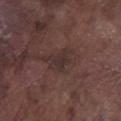Findings:
* follow-up · imaged on a skin check; not biopsied
* subject · male, aged 73–77
* imaging modality · total-body-photography crop, ~15 mm field of view
* lighting · white-light
* body site · the left lower leg